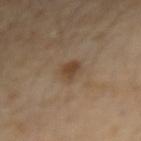No biopsy was performed on this lesion — it was imaged during a full skin examination and was not determined to be concerning. From the right forearm. Cropped from a total-body skin-imaging series; the visible field is about 15 mm. The patient is a female in their mid- to late 40s. About 2.5 mm across.